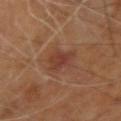lesion size: ~3 mm (longest diameter)
lighting: cross-polarized
automated lesion analysis: an average lesion color of about L≈38 a*≈23 b*≈28 (CIELAB), a lesion–skin lightness drop of about 7, and a lesion-to-skin contrast of about 6.5 (normalized; higher = more distinct)
subject: male, aged 68–72
image: 15 mm crop, total-body photography
body site: the right upper arm